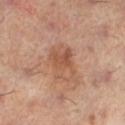The lesion was tiled from a total-body skin photograph and was not biopsied. About 4.5 mm across. The lesion is located on the left lower leg. Imaged with cross-polarized lighting. A 15 mm crop from a total-body photograph taken for skin-cancer surveillance. A female subject, about 60 years old.